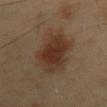body site — the mid back | acquisition — 15 mm crop, total-body photography | subject — male, about 40 years old.